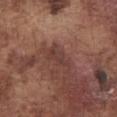| feature | finding |
|---|---|
| site | the chest |
| TBP lesion metrics | a lesion-to-skin contrast of about 6 (normalized; higher = more distinct) |
| subject | male, roughly 75 years of age |
| size | about 4 mm |
| lighting | white-light |
| image | 15 mm crop, total-body photography |